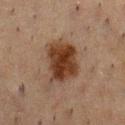Impression:
Part of a total-body skin-imaging series; this lesion was reviewed on a skin check and was not flagged for biopsy.
Context:
The subject is a male approximately 50 years of age. Imaged with cross-polarized lighting. A region of skin cropped from a whole-body photographic capture, roughly 15 mm wide. Automated tile analysis of the lesion measured an average lesion color of about L≈29 a*≈16 b*≈25 (CIELAB) and a lesion-to-skin contrast of about 12 (normalized; higher = more distinct). It also reported an automated nevus-likeness rating near 95 out of 100 and a detector confidence of about 100 out of 100 that the crop contains a lesion. Located on the chest. Measured at roughly 5 mm in maximum diameter.